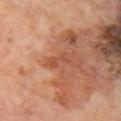The lesion was tiled from a total-body skin photograph and was not biopsied. The tile uses cross-polarized illumination. Cropped from a whole-body photographic skin survey; the tile spans about 15 mm. A male subject, in their mid-60s. From the right upper arm. Approximately 3 mm at its widest.